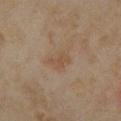follow-up = no biopsy performed (imaged during a skin exam)
body site = the right forearm
imaging modality = total-body-photography crop, ~15 mm field of view
patient = female, in their mid-30s
lesion size = ~2.5 mm (longest diameter)
image-analysis metrics = a mean CIELAB color near L≈47 a*≈16 b*≈29, a lesion–skin lightness drop of about 6, and a normalized border contrast of about 5.5; a classifier nevus-likeness of about 0/100 and a lesion-detection confidence of about 100/100
lighting = cross-polarized